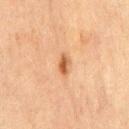Findings:
* biopsy status — catalogued during a skin exam; not biopsied
* patient — male, in their 70s
* image source — ~15 mm tile from a whole-body skin photo
* body site — the front of the torso
* automated metrics — an area of roughly 3 mm², a shape eccentricity near 0.85, and a shape-asymmetry score of about 0.3 (0 = symmetric)
* lesion diameter — ~2.5 mm (longest diameter)
* illumination — cross-polarized